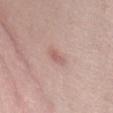Findings:
* follow-up: imaged on a skin check; not biopsied
* lighting: white-light illumination
* subject: female, roughly 40 years of age
* imaging modality: ~15 mm crop, total-body skin-cancer survey
* image-analysis metrics: border irregularity of about 3 on a 0–10 scale and peripheral color asymmetry of about 0; a detector confidence of about 100 out of 100 that the crop contains a lesion
* size: ≈2.5 mm
* anatomic site: the abdomen